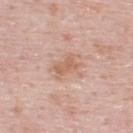Q: Was a biopsy performed?
A: no biopsy performed (imaged during a skin exam)
Q: Who is the patient?
A: male, approximately 50 years of age
Q: What is the anatomic site?
A: the upper back
Q: How large is the lesion?
A: about 3.5 mm
Q: What kind of image is this?
A: ~15 mm tile from a whole-body skin photo
Q: Illumination type?
A: white-light illumination